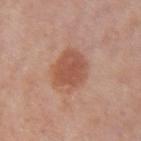Impression: Recorded during total-body skin imaging; not selected for excision or biopsy. Clinical summary: Captured under white-light illumination. A female subject approximately 60 years of age. The lesion's longest dimension is about 4.5 mm. A roughly 15 mm field-of-view crop from a total-body skin photograph. From the left upper arm.